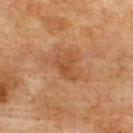biopsy_status: not biopsied; imaged during a skin examination
site: upper back
lighting: cross-polarized
image:
  source: total-body photography crop
  field_of_view_mm: 15
patient:
  sex: male
  age_approx: 75